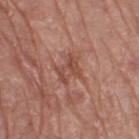Q: Was a biopsy performed?
A: no biopsy performed (imaged during a skin exam)
Q: Who is the patient?
A: male, about 70 years old
Q: What is the imaging modality?
A: 15 mm crop, total-body photography
Q: What is the anatomic site?
A: the right thigh